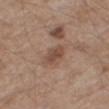The lesion was photographed on a routine skin check and not biopsied; there is no pathology result.
The lesion is on the right thigh.
The tile uses white-light illumination.
The lesion's longest dimension is about 3 mm.
Cropped from a whole-body photographic skin survey; the tile spans about 15 mm.
The patient is a male in their mid-60s.